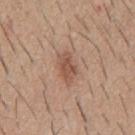Impression: This lesion was catalogued during total-body skin photography and was not selected for biopsy. Clinical summary: The lesion-visualizer software estimated a within-lesion color-variation index near 2.5/10. The lesion is on the mid back. A male patient, aged approximately 60. Longest diameter approximately 3.5 mm. Cropped from a whole-body photographic skin survey; the tile spans about 15 mm.